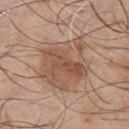{
  "biopsy_status": "not biopsied; imaged during a skin examination",
  "image": {
    "source": "total-body photography crop",
    "field_of_view_mm": 15
  },
  "lighting": "white-light",
  "automated_metrics": {
    "cielab_L": 54,
    "cielab_a": 19,
    "cielab_b": 28,
    "vs_skin_darker_L": 9.0,
    "vs_skin_contrast_norm": 6.5,
    "nevus_likeness_0_100": 50
  },
  "site": "chest",
  "patient": {
    "sex": "male",
    "age_approx": 45
  },
  "lesion_size": {
    "long_diameter_mm_approx": 6.5
  }
}